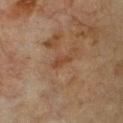Imaged during a routine full-body skin examination; the lesion was not biopsied and no histopathology is available. A 15 mm close-up extracted from a 3D total-body photography capture. The lesion's longest dimension is about 2.5 mm. This is a cross-polarized tile. A female patient, about 55 years old. The lesion is on the front of the torso.